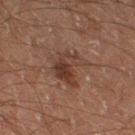workup: no biopsy performed (imaged during a skin exam); imaging modality: ~15 mm tile from a whole-body skin photo; lesion size: ≈4 mm; anatomic site: the right thigh; patient: male, aged around 60; tile lighting: cross-polarized.